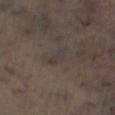No biopsy was performed on this lesion — it was imaged during a full skin examination and was not determined to be concerning.
Cropped from a total-body skin-imaging series; the visible field is about 15 mm.
Automated tile analysis of the lesion measured a mean CIELAB color near L≈36 a*≈9 b*≈15. And it measured a border-irregularity rating of about 4.5/10 and radial color variation of about 2.5. The analysis additionally found a nevus-likeness score of about 0/100 and a detector confidence of about 75 out of 100 that the crop contains a lesion.
The recorded lesion diameter is about 3.5 mm.
On the right lower leg.
This is a cross-polarized tile.